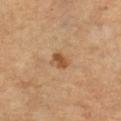The lesion was tiled from a total-body skin photograph and was not biopsied.
A female subject, approximately 70 years of age.
An algorithmic analysis of the crop reported a footprint of about 3.5 mm² and a symmetry-axis asymmetry near 0.25. The software also gave an average lesion color of about L≈49 a*≈21 b*≈35 (CIELAB), about 11 CIELAB-L* units darker than the surrounding skin, and a lesion-to-skin contrast of about 8 (normalized; higher = more distinct). The analysis additionally found a border-irregularity index near 2/10, a within-lesion color-variation index near 2/10, and peripheral color asymmetry of about 0.5.
From the leg.
A 15 mm close-up extracted from a 3D total-body photography capture.